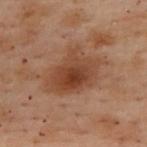| field | value |
|---|---|
| patient | female, roughly 55 years of age |
| imaging modality | total-body-photography crop, ~15 mm field of view |
| location | the upper back |
| size | ≈5.5 mm |
| tile lighting | cross-polarized |